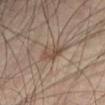- follow-up · imaged on a skin check; not biopsied
- illumination · cross-polarized illumination
- subject · male, in their 60s
- automated metrics · border irregularity of about 3.5 on a 0–10 scale and a within-lesion color-variation index near 4.5/10; a classifier nevus-likeness of about 5/100 and lesion-presence confidence of about 100/100
- anatomic site · the abdomen
- imaging modality · total-body-photography crop, ~15 mm field of view
- lesion size · about 3.5 mm